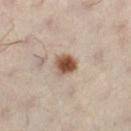workup=total-body-photography surveillance lesion; no biopsy